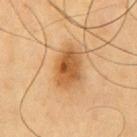This lesion was catalogued during total-body skin photography and was not selected for biopsy. From the chest. A male subject aged approximately 60. Cropped from a total-body skin-imaging series; the visible field is about 15 mm. Longest diameter approximately 5 mm. Automated tile analysis of the lesion measured an automated nevus-likeness rating near 95 out of 100 and a lesion-detection confidence of about 100/100. Imaged with cross-polarized lighting.